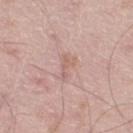Part of a total-body skin-imaging series; this lesion was reviewed on a skin check and was not flagged for biopsy.
From the right thigh.
Automated tile analysis of the lesion measured a footprint of about 4.5 mm², a shape eccentricity near 0.9, and a symmetry-axis asymmetry near 0.5. The software also gave a mean CIELAB color near L≈62 a*≈19 b*≈24, a lesion–skin lightness drop of about 6, and a normalized border contrast of about 4.5. And it measured a border-irregularity rating of about 5/10 and radial color variation of about 0.5.
Imaged with white-light lighting.
A roughly 15 mm field-of-view crop from a total-body skin photograph.
Measured at roughly 3.5 mm in maximum diameter.
A male subject in their 50s.